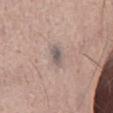Q: Was a biopsy performed?
A: catalogued during a skin exam; not biopsied
Q: What is the lesion's diameter?
A: ≈2.5 mm
Q: What did automated image analysis measure?
A: a footprint of about 3.5 mm² and an outline eccentricity of about 0.85 (0 = round, 1 = elongated); a lesion–skin lightness drop of about 10 and a lesion-to-skin contrast of about 8 (normalized; higher = more distinct)
Q: What kind of image is this?
A: 15 mm crop, total-body photography
Q: Who is the patient?
A: male, aged around 55